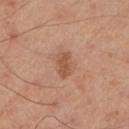{
  "biopsy_status": "not biopsied; imaged during a skin examination",
  "image": {
    "source": "total-body photography crop",
    "field_of_view_mm": 15
  },
  "patient": {
    "sex": "male",
    "age_approx": 50
  },
  "site": "left lower leg"
}